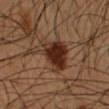<tbp_lesion>
<biopsy_status>not biopsied; imaged during a skin examination</biopsy_status>
<lesion_size>
  <long_diameter_mm_approx>6.0</long_diameter_mm_approx>
</lesion_size>
<image>
  <source>total-body photography crop</source>
  <field_of_view_mm>15</field_of_view_mm>
</image>
<lighting>cross-polarized</lighting>
<patient>
  <sex>male</sex>
  <age_approx>55</age_approx>
</patient>
<site>left forearm</site>
<automated_metrics>
  <area_mm2_approx>13.0</area_mm2_approx>
  <eccentricity>0.7</eccentricity>
  <shape_asymmetry>0.4</shape_asymmetry>
  <nevus_likeness_0_100>95</nevus_likeness_0_100>
  <lesion_detection_confidence_0_100>100</lesion_detection_confidence_0_100>
</automated_metrics>
</tbp_lesion>